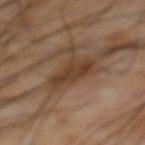The lesion was tiled from a total-body skin photograph and was not biopsied.
A 15 mm close-up extracted from a 3D total-body photography capture.
The lesion is located on the front of the torso.
A male patient, roughly 65 years of age.
The total-body-photography lesion software estimated an area of roughly 9.5 mm², a shape eccentricity near 0.75, and two-axis asymmetry of about 0.25.
The tile uses cross-polarized illumination.
The recorded lesion diameter is about 4.5 mm.